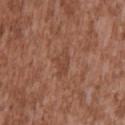notes = no biopsy performed (imaged during a skin exam) | subject = male, aged 43 to 47 | site = the upper back | image-analysis metrics = an average lesion color of about L≈44 a*≈22 b*≈29 (CIELAB), about 6 CIELAB-L* units darker than the surrounding skin, and a normalized border contrast of about 4.5 | acquisition = total-body-photography crop, ~15 mm field of view | diameter = ~4 mm (longest diameter).